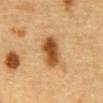Impression: Captured during whole-body skin photography for melanoma surveillance; the lesion was not biopsied. Acquisition and patient details: A close-up tile cropped from a whole-body skin photograph, about 15 mm across. This is a cross-polarized tile. The lesion's longest dimension is about 4 mm. The lesion is located on the abdomen. A male subject, in their mid- to late 80s or older.